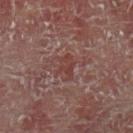follow-up — imaged on a skin check; not biopsied
TBP lesion metrics — a lesion area of about 4 mm², an eccentricity of roughly 0.7, and a symmetry-axis asymmetry near 0.45; a border-irregularity index near 5/10, a within-lesion color-variation index near 2/10, and a peripheral color-asymmetry measure near 1; a nevus-likeness score of about 0/100
tile lighting — cross-polarized illumination
subject — male, approximately 60 years of age
body site — the left lower leg
lesion size — ≈3 mm
imaging modality — total-body-photography crop, ~15 mm field of view